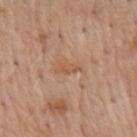Part of a total-body skin-imaging series; this lesion was reviewed on a skin check and was not flagged for biopsy. A male subject aged around 60. Cropped from a whole-body photographic skin survey; the tile spans about 15 mm. The lesion is located on the mid back. Captured under white-light illumination.